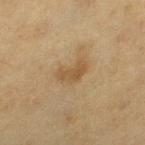Imaged during a routine full-body skin examination; the lesion was not biopsied and no histopathology is available. A 15 mm crop from a total-body photograph taken for skin-cancer surveillance. This is a cross-polarized tile. Measured at roughly 3 mm in maximum diameter. From the left thigh. A female subject, about 55 years old.